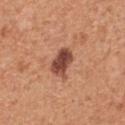{
  "biopsy_status": "not biopsied; imaged during a skin examination",
  "site": "chest",
  "patient": {
    "sex": "male",
    "age_approx": 55
  },
  "image": {
    "source": "total-body photography crop",
    "field_of_view_mm": 15
  },
  "lesion_size": {
    "long_diameter_mm_approx": 3.5
  },
  "lighting": "white-light"
}